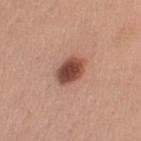Clinical impression:
Part of a total-body skin-imaging series; this lesion was reviewed on a skin check and was not flagged for biopsy.
Background:
This image is a 15 mm lesion crop taken from a total-body photograph. The subject is a female in their mid- to late 50s. Approximately 3.5 mm at its widest. Located on the left upper arm.